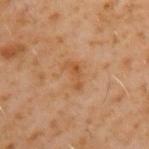Assessment: Part of a total-body skin-imaging series; this lesion was reviewed on a skin check and was not flagged for biopsy. Background: A male patient, in their 60s. Approximately 3 mm at its widest. A 15 mm crop from a total-body photograph taken for skin-cancer surveillance. Located on the back. The lesion-visualizer software estimated a symmetry-axis asymmetry near 0.45. The analysis additionally found a lesion-to-skin contrast of about 6 (normalized; higher = more distinct). The analysis additionally found a classifier nevus-likeness of about 0/100 and a detector confidence of about 100 out of 100 that the crop contains a lesion. Imaged with cross-polarized lighting.